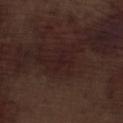Assessment: The lesion was tiled from a total-body skin photograph and was not biopsied. Acquisition and patient details: The total-body-photography lesion software estimated a lesion area of about 3 mm², an eccentricity of roughly 0.75, and two-axis asymmetry of about 0.6. And it measured a mean CIELAB color near L≈19 a*≈17 b*≈15, roughly 3 lightness units darker than nearby skin, and a normalized border contrast of about 5.5. The software also gave a border-irregularity rating of about 6.5/10, internal color variation of about 0 on a 0–10 scale, and peripheral color asymmetry of about 0. A close-up tile cropped from a whole-body skin photograph, about 15 mm across. Measured at roughly 2.5 mm in maximum diameter. Captured under white-light illumination. From the left lower leg. A male patient, aged 68–72.